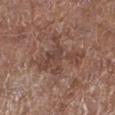workup — imaged on a skin check; not biopsied | location — the leg | tile lighting — white-light | diameter — about 5.5 mm | patient — female, about 80 years old | acquisition — ~15 mm crop, total-body skin-cancer survey | automated lesion analysis — a lesion area of about 15 mm² and a shape-asymmetry score of about 0.5 (0 = symmetric); a lesion color around L≈43 a*≈19 b*≈24 in CIELAB and a normalized lesion–skin contrast near 6; a border-irregularity index near 8.5/10, a color-variation rating of about 3/10, and peripheral color asymmetry of about 1; a nevus-likeness score of about 0/100.